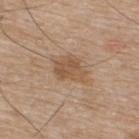This lesion was catalogued during total-body skin photography and was not selected for biopsy. The total-body-photography lesion software estimated an average lesion color of about L≈53 a*≈18 b*≈31 (CIELAB) and a normalized border contrast of about 6.5. And it measured a border-irregularity index near 3/10. This is a white-light tile. A male patient, aged around 75. Approximately 3.5 mm at its widest. On the back. A close-up tile cropped from a whole-body skin photograph, about 15 mm across.